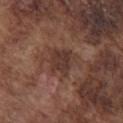Assessment:
The lesion was photographed on a routine skin check and not biopsied; there is no pathology result.
Image and clinical context:
The recorded lesion diameter is about 3 mm. The tile uses white-light illumination. A lesion tile, about 15 mm wide, cut from a 3D total-body photograph. The total-body-photography lesion software estimated a footprint of about 6 mm² and a shape eccentricity near 0.6. The analysis additionally found a lesion color around L≈34 a*≈19 b*≈22 in CIELAB, roughly 8 lightness units darker than nearby skin, and a lesion-to-skin contrast of about 7.5 (normalized; higher = more distinct). The software also gave a classifier nevus-likeness of about 5/100 and a lesion-detection confidence of about 100/100. The subject is a male in their mid- to late 70s. On the chest.